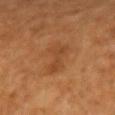| key | value |
|---|---|
| follow-up | no biopsy performed (imaged during a skin exam) |
| subject | female, approximately 50 years of age |
| illumination | cross-polarized illumination |
| image source | ~15 mm tile from a whole-body skin photo |
| body site | the right forearm |
| lesion size | about 4.5 mm |